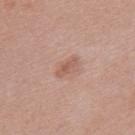biopsy status=imaged on a skin check; not biopsied | site=the upper back | imaging modality=~15 mm tile from a whole-body skin photo | patient=female, about 40 years old.